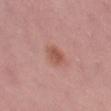Clinical impression: Part of a total-body skin-imaging series; this lesion was reviewed on a skin check and was not flagged for biopsy. Background: Longest diameter approximately 3 mm. A roughly 15 mm field-of-view crop from a total-body skin photograph. Automated tile analysis of the lesion measured an average lesion color of about L≈54 a*≈24 b*≈27 (CIELAB) and a normalized border contrast of about 7. And it measured an automated nevus-likeness rating near 95 out of 100 and a lesion-detection confidence of about 100/100. Imaged with white-light lighting. The patient is a female roughly 45 years of age. Located on the left thigh.